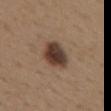The lesion was photographed on a routine skin check and not biopsied; there is no pathology result.
A close-up tile cropped from a whole-body skin photograph, about 15 mm across.
Located on the upper back.
About 4 mm across.
A female subject aged approximately 40.